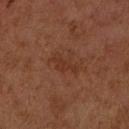Q: Was this lesion biopsied?
A: no biopsy performed (imaged during a skin exam)
Q: Lesion location?
A: the right upper arm
Q: What kind of image is this?
A: ~15 mm crop, total-body skin-cancer survey
Q: What are the patient's age and sex?
A: female, approximately 60 years of age